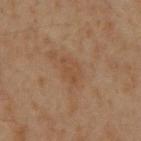Part of a total-body skin-imaging series; this lesion was reviewed on a skin check and was not flagged for biopsy.
A male subject, aged 58–62.
This image is a 15 mm lesion crop taken from a total-body photograph.
Longest diameter approximately 3.5 mm.
The lesion is on the mid back.
Captured under cross-polarized illumination.
The lesion-visualizer software estimated an average lesion color of about L≈43 a*≈17 b*≈29 (CIELAB) and roughly 5 lightness units darker than nearby skin.